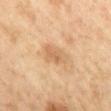Assessment: Part of a total-body skin-imaging series; this lesion was reviewed on a skin check and was not flagged for biopsy. Acquisition and patient details: The subject is a female aged 58 to 62. A 15 mm crop from a total-body photograph taken for skin-cancer surveillance. Captured under cross-polarized illumination. From the mid back.